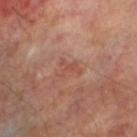Imaged during a routine full-body skin examination; the lesion was not biopsied and no histopathology is available. Measured at roughly 2.5 mm in maximum diameter. The total-body-photography lesion software estimated a symmetry-axis asymmetry near 0.6. And it measured a mean CIELAB color near L≈48 a*≈24 b*≈27, about 6 CIELAB-L* units darker than the surrounding skin, and a lesion-to-skin contrast of about 4.5 (normalized; higher = more distinct). And it measured a border-irregularity rating of about 8/10. The lesion is on the left lower leg. Imaged with cross-polarized lighting. A 15 mm close-up tile from a total-body photography series done for melanoma screening. The patient is a male roughly 70 years of age.